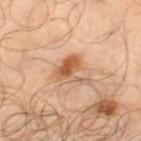Located on the right thigh.
A region of skin cropped from a whole-body photographic capture, roughly 15 mm wide.
Captured under cross-polarized illumination.
A male patient aged 63–67.
Automated tile analysis of the lesion measured a shape-asymmetry score of about 0.55 (0 = symmetric). The analysis additionally found a detector confidence of about 100 out of 100 that the crop contains a lesion.